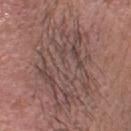Clinical impression:
Imaged during a routine full-body skin examination; the lesion was not biopsied and no histopathology is available.
Image and clinical context:
Cropped from a whole-body photographic skin survey; the tile spans about 15 mm. Automated tile analysis of the lesion measured a classifier nevus-likeness of about 0/100 and lesion-presence confidence of about 50/100. The patient is a female about 50 years old. Measured at roughly 9.5 mm in maximum diameter. From the head or neck.